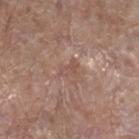No biopsy was performed on this lesion — it was imaged during a full skin examination and was not determined to be concerning.
A male subject aged approximately 45.
The lesion is located on the right lower leg.
Longest diameter approximately 2.5 mm.
Imaged with white-light lighting.
Cropped from a whole-body photographic skin survey; the tile spans about 15 mm.